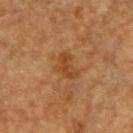The lesion-visualizer software estimated a border-irregularity index near 5/10. The analysis additionally found lesion-presence confidence of about 100/100. Measured at roughly 3.5 mm in maximum diameter. The subject is a male approximately 85 years of age or older. This is a cross-polarized tile. This image is a 15 mm lesion crop taken from a total-body photograph. Located on the head or neck.